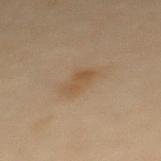Impression: The lesion was tiled from a total-body skin photograph and was not biopsied. Acquisition and patient details: The subject is a female approximately 60 years of age. Cropped from a total-body skin-imaging series; the visible field is about 15 mm. The lesion-visualizer software estimated a lesion area of about 4.5 mm², an eccentricity of roughly 0.75, and a symmetry-axis asymmetry near 0.3. The analysis additionally found an average lesion color of about L≈44 a*≈13 b*≈30 (CIELAB) and a normalized lesion–skin contrast near 6. Captured under cross-polarized illumination. Approximately 3 mm at its widest. Located on the upper back.